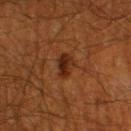Imaged during a routine full-body skin examination; the lesion was not biopsied and no histopathology is available.
A male patient aged around 60.
A region of skin cropped from a whole-body photographic capture, roughly 15 mm wide.
Located on the left thigh.